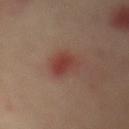Q: Was a biopsy performed?
A: no biopsy performed (imaged during a skin exam)
Q: What is the lesion's diameter?
A: ≈4.5 mm
Q: Lesion location?
A: the mid back
Q: What are the patient's age and sex?
A: female, aged 38–42
Q: What did automated image analysis measure?
A: an automated nevus-likeness rating near 45 out of 100 and lesion-presence confidence of about 100/100
Q: How was this image acquired?
A: 15 mm crop, total-body photography
Q: What lighting was used for the tile?
A: cross-polarized